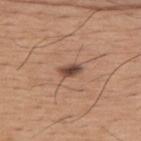No biopsy was performed on this lesion — it was imaged during a full skin examination and was not determined to be concerning.
The total-body-photography lesion software estimated a lesion color around L≈47 a*≈20 b*≈27 in CIELAB and a normalized border contrast of about 10.
A male subject in their mid-60s.
Captured under white-light illumination.
The lesion is located on the upper back.
Cropped from a total-body skin-imaging series; the visible field is about 15 mm.